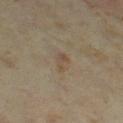{
  "biopsy_status": "not biopsied; imaged during a skin examination",
  "automated_metrics": {
    "area_mm2_approx": 2.5,
    "eccentricity": 0.9,
    "shape_asymmetry": 0.5,
    "nevus_likeness_0_100": 0,
    "lesion_detection_confidence_0_100": 100
  },
  "lighting": "cross-polarized",
  "lesion_size": {
    "long_diameter_mm_approx": 2.5
  },
  "image": {
    "source": "total-body photography crop",
    "field_of_view_mm": 15
  },
  "patient": {
    "sex": "female",
    "age_approx": 35
  },
  "site": "right thigh"
}